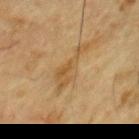Assessment:
Part of a total-body skin-imaging series; this lesion was reviewed on a skin check and was not flagged for biopsy.
Clinical summary:
This is a cross-polarized tile. A male subject about 85 years old or older. A region of skin cropped from a whole-body photographic capture, roughly 15 mm wide. On the chest. Longest diameter approximately 6 mm.